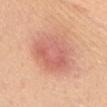| key | value |
|---|---|
| notes | total-body-photography surveillance lesion; no biopsy |
| image source | ~15 mm crop, total-body skin-cancer survey |
| size | ~6 mm (longest diameter) |
| site | the chest |
| lighting | white-light illumination |
| subject | female, in their mid- to late 40s |
| TBP lesion metrics | a nevus-likeness score of about 35/100 and a detector confidence of about 100 out of 100 that the crop contains a lesion |